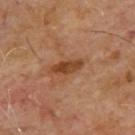Notes:
• workup · imaged on a skin check; not biopsied
• acquisition · ~15 mm crop, total-body skin-cancer survey
• location · the upper back
• patient · male, aged 63 to 67